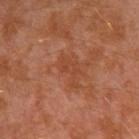{
  "biopsy_status": "not biopsied; imaged during a skin examination",
  "patient": {
    "sex": "male",
    "age_approx": 30
  },
  "site": "left arm",
  "image": {
    "source": "total-body photography crop",
    "field_of_view_mm": 15
  },
  "lesion_size": {
    "long_diameter_mm_approx": 3.5
  }
}